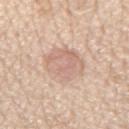{
  "biopsy_status": "not biopsied; imaged during a skin examination",
  "patient": {
    "sex": "male",
    "age_approx": 75
  },
  "site": "chest",
  "image": {
    "source": "total-body photography crop",
    "field_of_view_mm": 15
  }
}